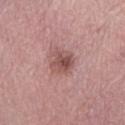Q: Was a biopsy performed?
A: no biopsy performed (imaged during a skin exam)
Q: Where on the body is the lesion?
A: the left lower leg
Q: How was this image acquired?
A: ~15 mm crop, total-body skin-cancer survey
Q: What is the lesion's diameter?
A: ~3 mm (longest diameter)
Q: What are the patient's age and sex?
A: female, aged approximately 65
Q: What did automated image analysis measure?
A: a lesion color around L≈51 a*≈23 b*≈22 in CIELAB, a lesion–skin lightness drop of about 11, and a normalized border contrast of about 7.5; border irregularity of about 2.5 on a 0–10 scale and a within-lesion color-variation index near 5/10
Q: How was the tile lit?
A: white-light illumination